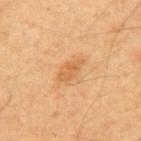Part of a total-body skin-imaging series; this lesion was reviewed on a skin check and was not flagged for biopsy. The subject is a male aged approximately 60. From the chest. A 15 mm close-up extracted from a 3D total-body photography capture.